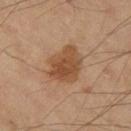<tbp_lesion>
  <biopsy_status>not biopsied; imaged during a skin examination</biopsy_status>
  <image>
    <source>total-body photography crop</source>
    <field_of_view_mm>15</field_of_view_mm>
  </image>
  <site>left thigh</site>
  <automated_metrics>
    <cielab_L>46</cielab_L>
    <cielab_a>19</cielab_a>
    <cielab_b>32</cielab_b>
    <vs_skin_darker_L>10.0</vs_skin_darker_L>
    <vs_skin_contrast_norm>8.0</vs_skin_contrast_norm>
    <border_irregularity_0_10>2.5</border_irregularity_0_10>
    <color_variation_0_10>4.0</color_variation_0_10>
    <nevus_likeness_0_100>85</nevus_likeness_0_100>
    <lesion_detection_confidence_0_100>100</lesion_detection_confidence_0_100>
  </automated_metrics>
  <patient>
    <sex>male</sex>
    <age_approx>70</age_approx>
  </patient>
  <lighting>cross-polarized</lighting>
</tbp_lesion>